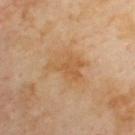biopsy_status: not biopsied; imaged during a skin examination
patient:
  sex: male
  age_approx: 70
image:
  source: total-body photography crop
  field_of_view_mm: 15
lighting: cross-polarized
site: upper back
lesion_size:
  long_diameter_mm_approx: 5.0